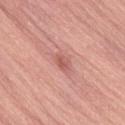Part of a total-body skin-imaging series; this lesion was reviewed on a skin check and was not flagged for biopsy. Approximately 2.5 mm at its widest. The subject is a female about 65 years old. A lesion tile, about 15 mm wide, cut from a 3D total-body photograph. The lesion is on the right thigh. Automated image analysis of the tile measured a symmetry-axis asymmetry near 0.2. And it measured a lesion color around L≈58 a*≈28 b*≈27 in CIELAB, about 9 CIELAB-L* units darker than the surrounding skin, and a normalized border contrast of about 6. And it measured a classifier nevus-likeness of about 5/100 and a detector confidence of about 100 out of 100 that the crop contains a lesion. This is a white-light tile.